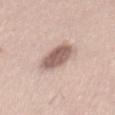Context: Cropped from a total-body skin-imaging series; the visible field is about 15 mm. The lesion is located on the back. A male patient, aged around 45.